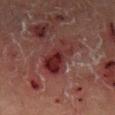Q: Is there a histopathology result?
A: total-body-photography surveillance lesion; no biopsy
Q: Who is the patient?
A: male, aged around 55
Q: What is the lesion's diameter?
A: about 5.5 mm
Q: Where on the body is the lesion?
A: the left lower leg
Q: How was the tile lit?
A: cross-polarized illumination
Q: What kind of image is this?
A: total-body-photography crop, ~15 mm field of view
Q: What did automated image analysis measure?
A: a mean CIELAB color near L≈26 a*≈25 b*≈19 and a lesion-to-skin contrast of about 10 (normalized; higher = more distinct); a nevus-likeness score of about 0/100 and a lesion-detection confidence of about 90/100